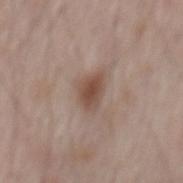The lesion was tiled from a total-body skin photograph and was not biopsied.
The lesion is located on the mid back.
The subject is a male about 65 years old.
A roughly 15 mm field-of-view crop from a total-body skin photograph.
The recorded lesion diameter is about 3.5 mm.